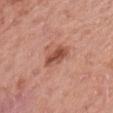<record>
  <biopsy_status>not biopsied; imaged during a skin examination</biopsy_status>
  <image>
    <source>total-body photography crop</source>
    <field_of_view_mm>15</field_of_view_mm>
  </image>
  <lesion_size>
    <long_diameter_mm_approx>3.5</long_diameter_mm_approx>
  </lesion_size>
  <site>right forearm</site>
  <lighting>white-light</lighting>
  <patient>
    <sex>female</sex>
    <age_approx>65</age_approx>
  </patient>
  <automated_metrics>
    <cielab_L>51</cielab_L>
    <cielab_a>27</cielab_a>
    <cielab_b>30</cielab_b>
    <vs_skin_darker_L>12.0</vs_skin_darker_L>
    <vs_skin_contrast_norm>8.5</vs_skin_contrast_norm>
    <color_variation_0_10>3.5</color_variation_0_10>
    <peripheral_color_asymmetry>1.5</peripheral_color_asymmetry>
  </automated_metrics>
</record>